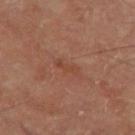The lesion was tiled from a total-body skin photograph and was not biopsied. A male subject, aged 68–72. A 15 mm crop from a total-body photograph taken for skin-cancer surveillance. Located on the left thigh. Captured under cross-polarized illumination. The lesion-visualizer software estimated an area of roughly 2.5 mm², an outline eccentricity of about 0.95 (0 = round, 1 = elongated), and a symmetry-axis asymmetry near 0.35. The analysis additionally found an average lesion color of about L≈42 a*≈24 b*≈30 (CIELAB), about 5 CIELAB-L* units darker than the surrounding skin, and a normalized lesion–skin contrast near 5.5. It also reported a border-irregularity index near 4/10 and peripheral color asymmetry of about 0.